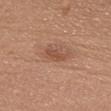Assessment: Recorded during total-body skin imaging; not selected for excision or biopsy. Acquisition and patient details: Automated tile analysis of the lesion measured a mean CIELAB color near L≈50 a*≈23 b*≈31. And it measured a border-irregularity index near 3/10 and radial color variation of about 1.5. The subject is a male aged approximately 30. This image is a 15 mm lesion crop taken from a total-body photograph. On the head or neck. This is a white-light tile.